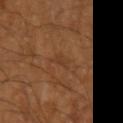Q: Was a biopsy performed?
A: total-body-photography surveillance lesion; no biopsy
Q: How was this image acquired?
A: ~15 mm crop, total-body skin-cancer survey
Q: How large is the lesion?
A: about 2.5 mm
Q: Who is the patient?
A: male, in their 60s
Q: Lesion location?
A: the arm